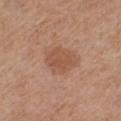<lesion>
  <biopsy_status>not biopsied; imaged during a skin examination</biopsy_status>
  <image>
    <source>total-body photography crop</source>
    <field_of_view_mm>15</field_of_view_mm>
  </image>
  <site>left thigh</site>
  <lesion_size>
    <long_diameter_mm_approx>4.5</long_diameter_mm_approx>
  </lesion_size>
  <patient>
    <sex>female</sex>
    <age_approx>55</age_approx>
  </patient>
</lesion>